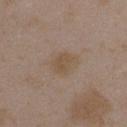Q: Was this lesion biopsied?
A: catalogued during a skin exam; not biopsied
Q: What kind of image is this?
A: total-body-photography crop, ~15 mm field of view
Q: Where on the body is the lesion?
A: the arm
Q: Patient demographics?
A: female, in their mid- to late 30s
Q: How large is the lesion?
A: ~3 mm (longest diameter)